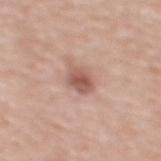workup=total-body-photography surveillance lesion; no biopsy
subject=female, aged around 40
automated lesion analysis=a footprint of about 5.5 mm², a shape eccentricity near 0.55, and two-axis asymmetry of about 0.1; border irregularity of about 1 on a 0–10 scale, internal color variation of about 3 on a 0–10 scale, and radial color variation of about 1; an automated nevus-likeness rating near 80 out of 100 and lesion-presence confidence of about 100/100
acquisition=15 mm crop, total-body photography
anatomic site=the mid back
lesion diameter=~3 mm (longest diameter)